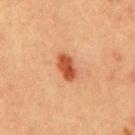Impression:
The lesion was photographed on a routine skin check and not biopsied; there is no pathology result.
Acquisition and patient details:
A 15 mm close-up extracted from a 3D total-body photography capture. Imaged with cross-polarized lighting. A male patient aged 38 to 42. The lesion is on the mid back.